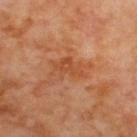Imaged during a routine full-body skin examination; the lesion was not biopsied and no histopathology is available.
A male subject, roughly 70 years of age.
The lesion-visualizer software estimated a nevus-likeness score of about 0/100 and a detector confidence of about 100 out of 100 that the crop contains a lesion.
A 15 mm crop from a total-body photograph taken for skin-cancer surveillance.
About 4 mm across.
Located on the upper back.